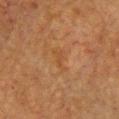follow-up: catalogued during a skin exam; not biopsied
subject: female, approximately 55 years of age
tile lighting: cross-polarized illumination
imaging modality: ~15 mm crop, total-body skin-cancer survey
size: ≈3.5 mm
anatomic site: the front of the torso
automated lesion analysis: a mean CIELAB color near L≈40 a*≈19 b*≈33, a lesion–skin lightness drop of about 4, and a lesion-to-skin contrast of about 4.5 (normalized; higher = more distinct)